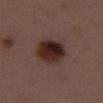Captured during whole-body skin photography for melanoma surveillance; the lesion was not biopsied.
This is a white-light tile.
A female patient, aged 48–52.
From the chest.
A 15 mm close-up tile from a total-body photography series done for melanoma screening.
Automated image analysis of the tile measured an outline eccentricity of about 0.55 (0 = round, 1 = elongated) and a symmetry-axis asymmetry near 0.15. It also reported an average lesion color of about L≈26 a*≈18 b*≈20 (CIELAB), about 14 CIELAB-L* units darker than the surrounding skin, and a normalized border contrast of about 13.5. The software also gave border irregularity of about 1.5 on a 0–10 scale and a color-variation rating of about 8/10.
The lesion's longest dimension is about 4.5 mm.